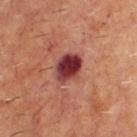Clinical impression:
The lesion was photographed on a routine skin check and not biopsied; there is no pathology result.
Acquisition and patient details:
An algorithmic analysis of the crop reported a normalized border contrast of about 13.5. And it measured a within-lesion color-variation index near 8.5/10 and peripheral color asymmetry of about 2.5. It also reported an automated nevus-likeness rating near 45 out of 100 and a detector confidence of about 100 out of 100 that the crop contains a lesion. On the upper back. This is a cross-polarized tile. Longest diameter approximately 4 mm. A 15 mm close-up tile from a total-body photography series done for melanoma screening. A male patient, aged 63–67.